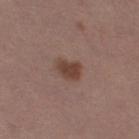Notes:
– notes: total-body-photography surveillance lesion; no biopsy
– body site: the right thigh
– image source: 15 mm crop, total-body photography
– lesion diameter: ~3 mm (longest diameter)
– subject: female, aged approximately 50